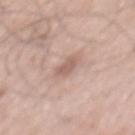Assessment:
The lesion was photographed on a routine skin check and not biopsied; there is no pathology result.
Background:
The subject is a male aged around 60. The total-body-photography lesion software estimated a border-irregularity index near 2.5/10, a within-lesion color-variation index near 1.5/10, and a peripheral color-asymmetry measure near 0.5. It also reported an automated nevus-likeness rating near 5 out of 100 and a detector confidence of about 100 out of 100 that the crop contains a lesion. A close-up tile cropped from a whole-body skin photograph, about 15 mm across. The lesion is located on the mid back. Imaged with white-light lighting.